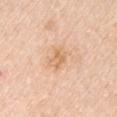{
  "biopsy_status": "not biopsied; imaged during a skin examination",
  "image": {
    "source": "total-body photography crop",
    "field_of_view_mm": 15
  },
  "lesion_size": {
    "long_diameter_mm_approx": 2.5
  },
  "patient": {
    "sex": "female",
    "age_approx": 45
  },
  "automated_metrics": {
    "area_mm2_approx": 4.0,
    "eccentricity": 0.7,
    "shape_asymmetry": 0.3,
    "vs_skin_darker_L": 8.0,
    "vs_skin_contrast_norm": 6.0,
    "nevus_likeness_0_100": 0,
    "lesion_detection_confidence_0_100": 100
  },
  "lighting": "white-light",
  "site": "right upper arm"
}